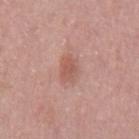| field | value |
|---|---|
| follow-up | catalogued during a skin exam; not biopsied |
| imaging modality | ~15 mm crop, total-body skin-cancer survey |
| body site | the mid back |
| image-analysis metrics | an area of roughly 5.5 mm², an outline eccentricity of about 0.65 (0 = round, 1 = elongated), and two-axis asymmetry of about 0.2 |
| patient | male, aged 53–57 |
| lesion diameter | ~3 mm (longest diameter) |